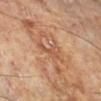notes: no biopsy performed (imaged during a skin exam); location: the right lower leg; patient: male, aged around 70; image: ~15 mm crop, total-body skin-cancer survey; lesion diameter: about 3.5 mm.